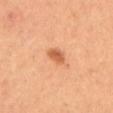– notes · imaged on a skin check; not biopsied
– imaging modality · 15 mm crop, total-body photography
– patient · female
– lesion diameter · ~2.5 mm (longest diameter)
– anatomic site · the abdomen